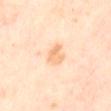Assessment: No biopsy was performed on this lesion — it was imaged during a full skin examination and was not determined to be concerning. Acquisition and patient details: The recorded lesion diameter is about 3 mm. A lesion tile, about 15 mm wide, cut from a 3D total-body photograph. A female subject, approximately 50 years of age. The lesion is located on the abdomen. Imaged with cross-polarized lighting.